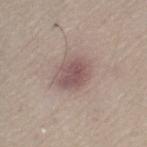Clinical impression: No biopsy was performed on this lesion — it was imaged during a full skin examination and was not determined to be concerning. Context: The lesion is located on the left thigh. Imaged with white-light lighting. The lesion's longest dimension is about 4.5 mm. A female patient, approximately 60 years of age. A lesion tile, about 15 mm wide, cut from a 3D total-body photograph.